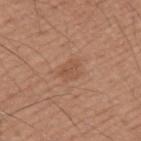Notes:
– follow-up: imaged on a skin check; not biopsied
– diameter: about 3 mm
– anatomic site: the back
– image-analysis metrics: an area of roughly 5 mm², a shape eccentricity near 0.7, and a shape-asymmetry score of about 0.3 (0 = symmetric); a detector confidence of about 100 out of 100 that the crop contains a lesion
– tile lighting: white-light
– image source: ~15 mm crop, total-body skin-cancer survey
– patient: male, roughly 20 years of age